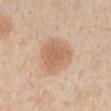follow-up = catalogued during a skin exam; not biopsied | image = 15 mm crop, total-body photography | body site = the right upper arm | illumination = white-light illumination | diameter = ~4.5 mm (longest diameter) | patient = male, approximately 60 years of age.